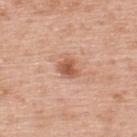Findings:
- lesion diameter: about 2.5 mm
- patient: male, aged around 60
- body site: the upper back
- lighting: white-light illumination
- image: 15 mm crop, total-body photography
- automated metrics: a footprint of about 4.5 mm², an eccentricity of roughly 0.55, and a symmetry-axis asymmetry near 0.25; a lesion color around L≈56 a*≈24 b*≈33 in CIELAB, roughly 12 lightness units darker than nearby skin, and a normalized lesion–skin contrast near 8; a nevus-likeness score of about 85/100